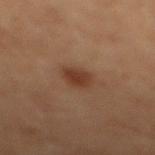Assessment: Captured during whole-body skin photography for melanoma surveillance; the lesion was not biopsied. Clinical summary: On the mid back. A male patient, aged approximately 70. The total-body-photography lesion software estimated an area of roughly 5 mm², an eccentricity of roughly 0.6, and a shape-asymmetry score of about 0.15 (0 = symmetric). The analysis additionally found a border-irregularity rating of about 1.5/10, a color-variation rating of about 1.5/10, and peripheral color asymmetry of about 0.5. And it measured a classifier nevus-likeness of about 90/100. A 15 mm crop from a total-body photograph taken for skin-cancer surveillance.